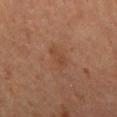Impression:
No biopsy was performed on this lesion — it was imaged during a full skin examination and was not determined to be concerning.
Clinical summary:
Approximately 3.5 mm at its widest. The subject is a male in their mid-60s. Cropped from a total-body skin-imaging series; the visible field is about 15 mm. The lesion is located on the mid back.